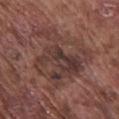The lesion was tiled from a total-body skin photograph and was not biopsied. Located on the front of the torso. The patient is a male aged around 75. Captured under white-light illumination. An algorithmic analysis of the crop reported a lesion area of about 30 mm². And it measured an average lesion color of about L≈37 a*≈19 b*≈21 (CIELAB) and a lesion-to-skin contrast of about 7 (normalized; higher = more distinct). This image is a 15 mm lesion crop taken from a total-body photograph. About 8.5 mm across.